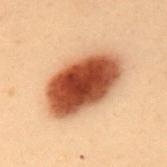<lesion>
<biopsy_status>not biopsied; imaged during a skin examination</biopsy_status>
<patient>
  <sex>female</sex>
  <age_approx>40</age_approx>
</patient>
<image>
  <source>total-body photography crop</source>
  <field_of_view_mm>15</field_of_view_mm>
</image>
<lesion_size>
  <long_diameter_mm_approx>8.5</long_diameter_mm_approx>
</lesion_size>
<site>back</site>
<lighting>cross-polarized</lighting>
</lesion>